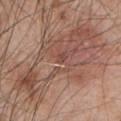Recorded during total-body skin imaging; not selected for excision or biopsy. A male subject about 45 years old. Captured under white-light illumination. This image is a 15 mm lesion crop taken from a total-body photograph. The lesion is on the left upper arm.